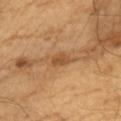Captured during whole-body skin photography for melanoma surveillance; the lesion was not biopsied. This is a cross-polarized tile. A male subject roughly 60 years of age. The lesion is on the chest. Measured at roughly 2.5 mm in maximum diameter. A lesion tile, about 15 mm wide, cut from a 3D total-body photograph. Automated image analysis of the tile measured a normalized lesion–skin contrast near 7.